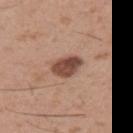No biopsy was performed on this lesion — it was imaged during a full skin examination and was not determined to be concerning.
A lesion tile, about 15 mm wide, cut from a 3D total-body photograph.
Imaged with white-light lighting.
Located on the left upper arm.
The subject is a male approximately 30 years of age.